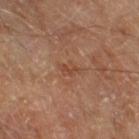Findings:
• biopsy status: imaged on a skin check; not biopsied
• image-analysis metrics: an eccentricity of roughly 0.85 and two-axis asymmetry of about 0.35; a lesion color around L≈44 a*≈22 b*≈30 in CIELAB, a lesion–skin lightness drop of about 6, and a normalized border contrast of about 5; a border-irregularity index near 3.5/10, a color-variation rating of about 1/10, and a peripheral color-asymmetry measure near 0; a nevus-likeness score of about 0/100 and a detector confidence of about 100 out of 100 that the crop contains a lesion
• diameter: ~2.5 mm (longest diameter)
• location: the right thigh
• imaging modality: 15 mm crop, total-body photography
• tile lighting: cross-polarized
• patient: male, aged 68 to 72